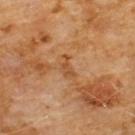notes: catalogued during a skin exam; not biopsied | size: ~3 mm (longest diameter) | acquisition: 15 mm crop, total-body photography | subject: male, aged 58–62 | tile lighting: cross-polarized | body site: the chest.